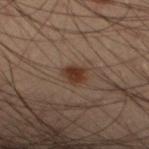No biopsy was performed on this lesion — it was imaged during a full skin examination and was not determined to be concerning. The tile uses cross-polarized illumination. From the left lower leg. Automated image analysis of the tile measured two-axis asymmetry of about 0.2. And it measured a lesion color around L≈27 a*≈15 b*≈22 in CIELAB, a lesion–skin lightness drop of about 9, and a normalized lesion–skin contrast near 10. The analysis additionally found border irregularity of about 1.5 on a 0–10 scale, internal color variation of about 1.5 on a 0–10 scale, and a peripheral color-asymmetry measure near 0.5. It also reported a classifier nevus-likeness of about 95/100. The lesion's longest dimension is about 2.5 mm. The subject is a male aged around 55. A roughly 15 mm field-of-view crop from a total-body skin photograph.